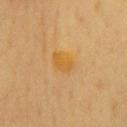Captured during whole-body skin photography for melanoma surveillance; the lesion was not biopsied.
A male subject, about 65 years old.
Measured at roughly 3 mm in maximum diameter.
A roughly 15 mm field-of-view crop from a total-body skin photograph.
Imaged with cross-polarized lighting.
From the chest.
The total-body-photography lesion software estimated a lesion area of about 6.5 mm² and an outline eccentricity of about 0.6 (0 = round, 1 = elongated). The analysis additionally found a nevus-likeness score of about 10/100 and lesion-presence confidence of about 100/100.